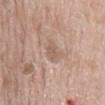Part of a total-body skin-imaging series; this lesion was reviewed on a skin check and was not flagged for biopsy. Cropped from a whole-body photographic skin survey; the tile spans about 15 mm. This is a white-light tile. Automated tile analysis of the lesion measured a lesion area of about 3 mm² and a shape eccentricity near 0.9. The software also gave a lesion color around L≈58 a*≈16 b*≈27 in CIELAB, roughly 7 lightness units darker than nearby skin, and a normalized lesion–skin contrast near 5. The analysis additionally found a border-irregularity rating of about 2.5/10. The software also gave an automated nevus-likeness rating near 0 out of 100. Approximately 3 mm at its widest. The subject is a male roughly 65 years of age. Located on the mid back.